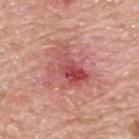Findings:
- workup — no biopsy performed (imaged during a skin exam)
- image — 15 mm crop, total-body photography
- site — the upper back
- automated metrics — a border-irregularity index near 7/10 and a color-variation rating of about 10/10
- size — ≈5 mm
- tile lighting — white-light
- patient — male, in their 80s